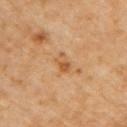Assessment: No biopsy was performed on this lesion — it was imaged during a full skin examination and was not determined to be concerning. Acquisition and patient details: Longest diameter approximately 3 mm. Imaged with cross-polarized lighting. Automated image analysis of the tile measured a mean CIELAB color near L≈54 a*≈21 b*≈39, roughly 8 lightness units darker than nearby skin, and a normalized lesion–skin contrast near 6.5. The software also gave a classifier nevus-likeness of about 0/100. From the upper back. This image is a 15 mm lesion crop taken from a total-body photograph. A male patient, aged approximately 60.